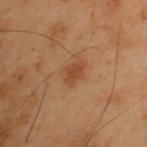Automated image analysis of the tile measured a lesion area of about 3.5 mm², a shape eccentricity near 0.65, and a shape-asymmetry score of about 0.35 (0 = symmetric). The software also gave a border-irregularity rating of about 3.5/10, internal color variation of about 1 on a 0–10 scale, and peripheral color asymmetry of about 0.5. The analysis additionally found a classifier nevus-likeness of about 65/100 and a detector confidence of about 100 out of 100 that the crop contains a lesion. The subject is a male aged 53 to 57. The lesion is located on the upper back. This is a cross-polarized tile. The recorded lesion diameter is about 2.5 mm. A region of skin cropped from a whole-body photographic capture, roughly 15 mm wide.